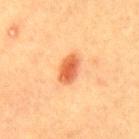Imaged during a routine full-body skin examination; the lesion was not biopsied and no histopathology is available. The subject is a male about 40 years old. A 15 mm close-up tile from a total-body photography series done for melanoma screening. About 3.5 mm across. The total-body-photography lesion software estimated a nevus-likeness score of about 100/100 and a detector confidence of about 100 out of 100 that the crop contains a lesion. The lesion is located on the mid back.